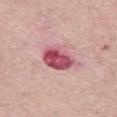Clinical impression:
Recorded during total-body skin imaging; not selected for excision or biopsy.
Acquisition and patient details:
The lesion is on the leg. Automated image analysis of the tile measured an outline eccentricity of about 0.55 (0 = round, 1 = elongated). And it measured border irregularity of about 2 on a 0–10 scale and internal color variation of about 9 on a 0–10 scale. The tile uses white-light illumination. A female subject, aged around 65. A roughly 15 mm field-of-view crop from a total-body skin photograph. The lesion's longest dimension is about 4.5 mm.